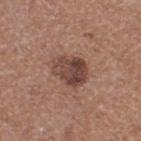<record>
  <biopsy_status>not biopsied; imaged during a skin examination</biopsy_status>
  <site>right thigh</site>
  <image>
    <source>total-body photography crop</source>
    <field_of_view_mm>15</field_of_view_mm>
  </image>
  <patient>
    <sex>female</sex>
    <age_approx>40</age_approx>
  </patient>
  <automated_metrics>
    <eccentricity>0.65</eccentricity>
    <shape_asymmetry>0.25</shape_asymmetry>
    <cielab_L>43</cielab_L>
    <cielab_a>21</cielab_a>
    <cielab_b>23</cielab_b>
    <vs_skin_contrast_norm>9.5</vs_skin_contrast_norm>
    <nevus_likeness_0_100>10</nevus_likeness_0_100>
    <lesion_detection_confidence_0_100>100</lesion_detection_confidence_0_100>
  </automated_metrics>
  <lighting>white-light</lighting>
  <lesion_size>
    <long_diameter_mm_approx>4.0</long_diameter_mm_approx>
  </lesion_size>
</record>